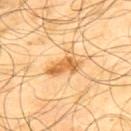workup = no biopsy performed (imaged during a skin exam)
lesion diameter = ~4.5 mm (longest diameter)
automated lesion analysis = a lesion color around L≈63 a*≈22 b*≈45 in CIELAB and a normalized lesion–skin contrast near 8; a border-irregularity rating of about 4.5/10 and radial color variation of about 1; an automated nevus-likeness rating near 35 out of 100 and lesion-presence confidence of about 100/100
body site = the back
subject = male, about 65 years old
image source = ~15 mm tile from a whole-body skin photo
lighting = cross-polarized